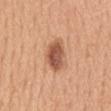Imaged during a routine full-body skin examination; the lesion was not biopsied and no histopathology is available.
On the mid back.
The patient is a male in their 50s.
The tile uses white-light illumination.
Cropped from a whole-body photographic skin survey; the tile spans about 15 mm.